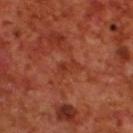Q: Is there a histopathology result?
A: catalogued during a skin exam; not biopsied
Q: What is the anatomic site?
A: the upper back
Q: What is the imaging modality?
A: ~15 mm tile from a whole-body skin photo
Q: Automated lesion metrics?
A: a border-irregularity index near 4.5/10, internal color variation of about 0.5 on a 0–10 scale, and peripheral color asymmetry of about 0
Q: How was the tile lit?
A: cross-polarized
Q: How large is the lesion?
A: about 2.5 mm
Q: What are the patient's age and sex?
A: male, about 70 years old